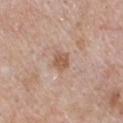Q: Is there a histopathology result?
A: catalogued during a skin exam; not biopsied
Q: Where on the body is the lesion?
A: the chest
Q: What is the imaging modality?
A: ~15 mm crop, total-body skin-cancer survey
Q: What lighting was used for the tile?
A: white-light illumination
Q: Who is the patient?
A: male, in their mid-60s